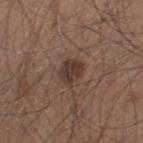biopsy status: catalogued during a skin exam; not biopsied
acquisition: total-body-photography crop, ~15 mm field of view
tile lighting: white-light illumination
lesion size: ≈3 mm
automated metrics: an average lesion color of about L≈36 a*≈17 b*≈22 (CIELAB), a lesion–skin lightness drop of about 10, and a normalized lesion–skin contrast near 8.5; a border-irregularity rating of about 1.5/10, a color-variation rating of about 2/10, and a peripheral color-asymmetry measure near 1
subject: male, about 45 years old
location: the left thigh